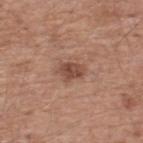Assessment:
This lesion was catalogued during total-body skin photography and was not selected for biopsy.
Clinical summary:
Approximately 3 mm at its widest. The tile uses white-light illumination. The lesion is on the back. The subject is a male approximately 55 years of age. Automated image analysis of the tile measured a footprint of about 5 mm², an outline eccentricity of about 0.7 (0 = round, 1 = elongated), and a symmetry-axis asymmetry near 0.35. The software also gave roughly 11 lightness units darker than nearby skin. And it measured a border-irregularity rating of about 3.5/10 and radial color variation of about 1. A close-up tile cropped from a whole-body skin photograph, about 15 mm across.